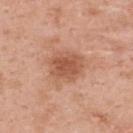{"biopsy_status": "not biopsied; imaged during a skin examination", "patient": {"sex": "female", "age_approx": 50}, "lighting": "white-light", "automated_metrics": {"area_mm2_approx": 8.5, "shape_asymmetry": 0.15, "cielab_L": 54, "cielab_a": 25, "cielab_b": 33, "vs_skin_darker_L": 11.0, "border_irregularity_0_10": 2.0, "color_variation_0_10": 2.5}, "image": {"source": "total-body photography crop", "field_of_view_mm": 15}, "site": "upper back", "lesion_size": {"long_diameter_mm_approx": 3.5}}